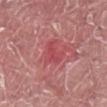The lesion was tiled from a total-body skin photograph and was not biopsied.
This image is a 15 mm lesion crop taken from a total-body photograph.
A male patient, in their 40s.
The lesion is located on the front of the torso.
The lesion's longest dimension is about 2.5 mm.
Imaged with white-light lighting.